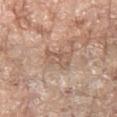Background:
A female subject aged around 75. This image is a 15 mm lesion crop taken from a total-body photograph. The tile uses white-light illumination. Located on the right forearm. The recorded lesion diameter is about 3.5 mm. Automated image analysis of the tile measured an average lesion color of about L≈58 a*≈17 b*≈28 (CIELAB), about 8 CIELAB-L* units darker than the surrounding skin, and a normalized border contrast of about 5. And it measured a color-variation rating of about 3.5/10 and a peripheral color-asymmetry measure near 1.5. The analysis additionally found a nevus-likeness score of about 0/100 and lesion-presence confidence of about 80/100.